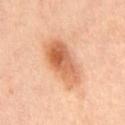- image-analysis metrics: a lesion–skin lightness drop of about 13 and a normalized border contrast of about 8; a nevus-likeness score of about 85/100 and a detector confidence of about 100 out of 100 that the crop contains a lesion
- lesion size: about 7 mm
- site: the mid back
- illumination: cross-polarized illumination
- image: total-body-photography crop, ~15 mm field of view